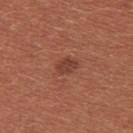This lesion was catalogued during total-body skin photography and was not selected for biopsy.
Cropped from a whole-body photographic skin survey; the tile spans about 15 mm.
On the chest.
A female subject approximately 35 years of age.